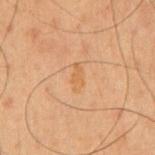The lesion was tiled from a total-body skin photograph and was not biopsied.
From the abdomen.
A male subject aged around 50.
The tile uses cross-polarized illumination.
An algorithmic analysis of the crop reported an average lesion color of about L≈46 a*≈17 b*≈32 (CIELAB), roughly 4 lightness units darker than nearby skin, and a normalized border contrast of about 5. And it measured a border-irregularity index near 3.5/10 and internal color variation of about 0.5 on a 0–10 scale.
A lesion tile, about 15 mm wide, cut from a 3D total-body photograph.